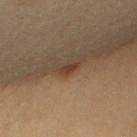Case summary:
* biopsy status — no biopsy performed (imaged during a skin exam)
* location — the upper back
* acquisition — ~15 mm crop, total-body skin-cancer survey
* diameter — about 2 mm
* subject — female, aged approximately 70
* image-analysis metrics — an average lesion color of about L≈39 a*≈18 b*≈30 (CIELAB), roughly 9 lightness units darker than nearby skin, and a normalized lesion–skin contrast near 8; a color-variation rating of about 0/10 and radial color variation of about 0; an automated nevus-likeness rating near 80 out of 100 and lesion-presence confidence of about 100/100